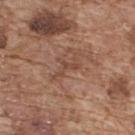No biopsy was performed on this lesion — it was imaged during a full skin examination and was not determined to be concerning.
An algorithmic analysis of the crop reported an area of roughly 3 mm², an eccentricity of roughly 0.9, and a symmetry-axis asymmetry near 0.75.
From the upper back.
A 15 mm close-up extracted from a 3D total-body photography capture.
A male patient, in their mid-70s.